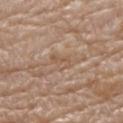The lesion was tiled from a total-body skin photograph and was not biopsied. About 2.5 mm across. An algorithmic analysis of the crop reported an area of roughly 4 mm² and a shape-asymmetry score of about 0.35 (0 = symmetric). The software also gave a lesion color around L≈55 a*≈16 b*≈30 in CIELAB, roughly 6 lightness units darker than nearby skin, and a normalized border contrast of about 5. Cropped from a total-body skin-imaging series; the visible field is about 15 mm. A male subject aged 78 to 82. From the back.